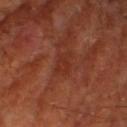follow-up: total-body-photography surveillance lesion; no biopsy | tile lighting: cross-polarized | subject: male, about 65 years old | image-analysis metrics: a mean CIELAB color near L≈30 a*≈27 b*≈29, a lesion–skin lightness drop of about 5, and a lesion-to-skin contrast of about 5 (normalized; higher = more distinct); border irregularity of about 3.5 on a 0–10 scale, a within-lesion color-variation index near 1.5/10, and radial color variation of about 0.5; a classifier nevus-likeness of about 0/100 and lesion-presence confidence of about 95/100 | image source: total-body-photography crop, ~15 mm field of view | anatomic site: the right lower leg.